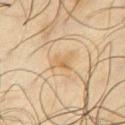biopsy status — catalogued during a skin exam; not biopsied
imaging modality — 15 mm crop, total-body photography
illumination — cross-polarized
anatomic site — the chest
subject — male, approximately 65 years of age
image-analysis metrics — a lesion color around L≈61 a*≈15 b*≈38 in CIELAB, about 7 CIELAB-L* units darker than the surrounding skin, and a normalized border contrast of about 5.5; an automated nevus-likeness rating near 5 out of 100
lesion diameter — ~3 mm (longest diameter)